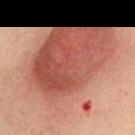Located on the chest.
The patient is a female aged approximately 70.
A 15 mm close-up tile from a total-body photography series done for melanoma screening.